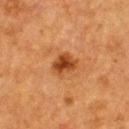biopsy status: catalogued during a skin exam; not biopsied | site: the upper back | diameter: ≈3 mm | image: ~15 mm tile from a whole-body skin photo | patient: female, aged 53 to 57 | automated metrics: a lesion area of about 6 mm² and a shape-asymmetry score of about 0.15 (0 = symmetric); an average lesion color of about L≈38 a*≈24 b*≈36 (CIELAB), a lesion–skin lightness drop of about 11, and a normalized border contrast of about 9.5.